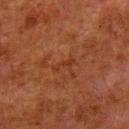<case>
  <biopsy_status>not biopsied; imaged during a skin examination</biopsy_status>
  <site>left lower leg</site>
  <image>
    <source>total-body photography crop</source>
    <field_of_view_mm>15</field_of_view_mm>
  </image>
  <patient>
    <sex>male</sex>
    <age_approx>80</age_approx>
  </patient>
  <automated_metrics>
    <eccentricity>0.95</eccentricity>
    <shape_asymmetry>0.4</shape_asymmetry>
    <cielab_L>28</cielab_L>
    <cielab_a>22</cielab_a>
    <cielab_b>28</cielab_b>
    <vs_skin_darker_L>4.0</vs_skin_darker_L>
    <vs_skin_contrast_norm>5.0</vs_skin_contrast_norm>
    <border_irregularity_0_10>5.0</border_irregularity_0_10>
    <color_variation_0_10>0.0</color_variation_0_10>
    <peripheral_color_asymmetry>0.0</peripheral_color_asymmetry>
  </automated_metrics>
</case>